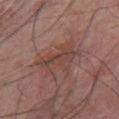Findings:
• workup · total-body-photography surveillance lesion; no biopsy
• lesion diameter · ≈6 mm
• tile lighting · white-light
• subject · male, in their 70s
• anatomic site · the chest
• automated metrics · a lesion area of about 14 mm², an eccentricity of roughly 0.85, and two-axis asymmetry of about 0.4; an average lesion color of about L≈43 a*≈20 b*≈23 (CIELAB), roughly 7 lightness units darker than nearby skin, and a normalized lesion–skin contrast near 6; a border-irregularity rating of about 5.5/10, a color-variation rating of about 4.5/10, and radial color variation of about 1.5; a classifier nevus-likeness of about 0/100
• image · total-body-photography crop, ~15 mm field of view